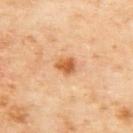Q: How was the tile lit?
A: cross-polarized illumination
Q: What kind of image is this?
A: ~15 mm tile from a whole-body skin photo
Q: Patient demographics?
A: female, aged around 60
Q: Where on the body is the lesion?
A: the upper back
Q: What did automated image analysis measure?
A: an area of roughly 4.5 mm², a shape eccentricity near 0.6, and two-axis asymmetry of about 0.2; an automated nevus-likeness rating near 90 out of 100
Q: What is the lesion's diameter?
A: about 3 mm